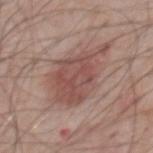Impression:
The lesion was tiled from a total-body skin photograph and was not biopsied.
Acquisition and patient details:
Imaged with white-light lighting. A 15 mm close-up extracted from a 3D total-body photography capture. Longest diameter approximately 8 mm. From the chest. The patient is a male aged 68–72. The total-body-photography lesion software estimated an average lesion color of about L≈50 a*≈21 b*≈23 (CIELAB) and a normalized lesion–skin contrast near 7. The analysis additionally found a border-irregularity rating of about 5/10 and internal color variation of about 3.5 on a 0–10 scale.